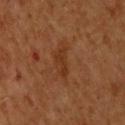biopsy status — no biopsy performed (imaged during a skin exam) | body site — the upper back | image — ~15 mm tile from a whole-body skin photo | subject — male, aged 63 to 67 | lesion diameter — ≈3.5 mm | lighting — cross-polarized.